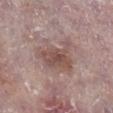Impression: This lesion was catalogued during total-body skin photography and was not selected for biopsy. Clinical summary: The tile uses white-light illumination. The total-body-photography lesion software estimated an area of roughly 17 mm², a shape eccentricity near 0.65, and two-axis asymmetry of about 0.4. The software also gave a lesion color around L≈52 a*≈18 b*≈21 in CIELAB, about 9 CIELAB-L* units darker than the surrounding skin, and a lesion-to-skin contrast of about 6.5 (normalized; higher = more distinct). The analysis additionally found a border-irregularity index near 5.5/10, internal color variation of about 4 on a 0–10 scale, and peripheral color asymmetry of about 1. It also reported an automated nevus-likeness rating near 55 out of 100 and a detector confidence of about 100 out of 100 that the crop contains a lesion. About 6 mm across. Cropped from a whole-body photographic skin survey; the tile spans about 15 mm. A male patient aged 73 to 77. The lesion is on the right lower leg.